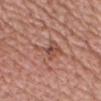<tbp_lesion>
  <biopsy_status>not biopsied; imaged during a skin examination</biopsy_status>
  <image>
    <source>total-body photography crop</source>
    <field_of_view_mm>15</field_of_view_mm>
  </image>
  <site>chest</site>
  <lighting>white-light</lighting>
  <automated_metrics>
    <area_mm2_approx>7.0</area_mm2_approx>
    <eccentricity>0.8</eccentricity>
    <cielab_L>50</cielab_L>
    <cielab_a>23</cielab_a>
    <cielab_b>27</cielab_b>
    <vs_skin_darker_L>8.0</vs_skin_darker_L>
    <vs_skin_contrast_norm>6.0</vs_skin_contrast_norm>
    <border_irregularity_0_10>7.0</border_irregularity_0_10>
    <color_variation_0_10>5.0</color_variation_0_10>
    <peripheral_color_asymmetry>1.5</peripheral_color_asymmetry>
  </automated_metrics>
  <patient>
    <sex>male</sex>
    <age_approx>60</age_approx>
  </patient>
</tbp_lesion>